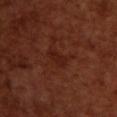Findings:
• biopsy status — imaged on a skin check; not biopsied
• automated lesion analysis — a mean CIELAB color near L≈22 a*≈24 b*≈26 and roughly 5 lightness units darker than nearby skin; a border-irregularity rating of about 3.5/10 and a within-lesion color-variation index near 0.5/10
• patient — female, approximately 55 years of age
• body site — the upper back
• lighting — cross-polarized illumination
• image source — ~15 mm tile from a whole-body skin photo
• diameter — about 3 mm